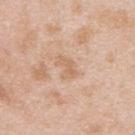<case>
<biopsy_status>not biopsied; imaged during a skin examination</biopsy_status>
<image>
  <source>total-body photography crop</source>
  <field_of_view_mm>15</field_of_view_mm>
</image>
<site>upper back</site>
<patient>
  <sex>male</sex>
  <age_approx>25</age_approx>
</patient>
<automated_metrics>
  <eccentricity>0.8</eccentricity>
  <cielab_L>65</cielab_L>
  <cielab_a>19</cielab_a>
  <cielab_b>33</cielab_b>
  <vs_skin_contrast_norm>5.0</vs_skin_contrast_norm>
  <nevus_likeness_0_100>0</nevus_likeness_0_100>
  <lesion_detection_confidence_0_100>100</lesion_detection_confidence_0_100>
</automated_metrics>
</case>